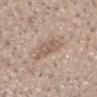{
  "biopsy_status": "not biopsied; imaged during a skin examination",
  "lesion_size": {
    "long_diameter_mm_approx": 3.0
  },
  "patient": {
    "sex": "male",
    "age_approx": 30
  },
  "site": "head or neck",
  "image": {
    "source": "total-body photography crop",
    "field_of_view_mm": 15
  }
}